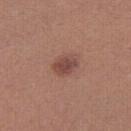Impression:
Part of a total-body skin-imaging series; this lesion was reviewed on a skin check and was not flagged for biopsy.
Acquisition and patient details:
Captured under white-light illumination. A roughly 15 mm field-of-view crop from a total-body skin photograph. A female patient, roughly 20 years of age. The lesion is on the right thigh.